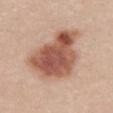Assessment:
No biopsy was performed on this lesion — it was imaged during a full skin examination and was not determined to be concerning.
Image and clinical context:
The lesion is located on the mid back. Cropped from a whole-body photographic skin survey; the tile spans about 15 mm. This is a white-light tile. The lesion's longest dimension is about 8 mm. The patient is a female about 20 years old. The lesion-visualizer software estimated a mean CIELAB color near L≈55 a*≈23 b*≈29, roughly 16 lightness units darker than nearby skin, and a lesion-to-skin contrast of about 10 (normalized; higher = more distinct).